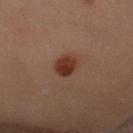biopsy status=catalogued during a skin exam; not biopsied | automated metrics=a lesion area of about 6 mm²; a nevus-likeness score of about 100/100 and lesion-presence confidence of about 100/100 | lighting=cross-polarized illumination | imaging modality=~15 mm crop, total-body skin-cancer survey | site=the chest | diameter=about 3 mm | subject=male, about 65 years old.